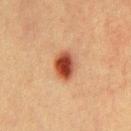Notes:
• biopsy status — total-body-photography surveillance lesion; no biopsy
• tile lighting — cross-polarized illumination
• acquisition — total-body-photography crop, ~15 mm field of view
• size — about 3.5 mm
• subject — male, about 40 years old
• site — the chest